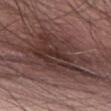{
  "biopsy_status": "not biopsied; imaged during a skin examination"
}